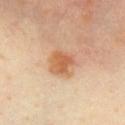biopsy status: imaged on a skin check; not biopsied | lighting: cross-polarized illumination | acquisition: ~15 mm crop, total-body skin-cancer survey | subject: female, in their mid-40s | image-analysis metrics: a mean CIELAB color near L≈59 a*≈22 b*≈36, about 10 CIELAB-L* units darker than the surrounding skin, and a lesion-to-skin contrast of about 7.5 (normalized; higher = more distinct); a border-irregularity index near 3/10 and a within-lesion color-variation index near 4.5/10; a lesion-detection confidence of about 100/100 | site: the chest.